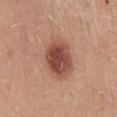Q: Was a biopsy performed?
A: imaged on a skin check; not biopsied
Q: What are the patient's age and sex?
A: male, aged around 25
Q: Lesion size?
A: ≈5 mm
Q: How was the tile lit?
A: white-light illumination
Q: Automated lesion metrics?
A: an average lesion color of about L≈49 a*≈24 b*≈27 (CIELAB), about 14 CIELAB-L* units darker than the surrounding skin, and a normalized lesion–skin contrast near 9.5; a border-irregularity index near 1.5/10, a within-lesion color-variation index near 5.5/10, and a peripheral color-asymmetry measure near 1.5; a nevus-likeness score of about 100/100
Q: Where on the body is the lesion?
A: the mid back
Q: What is the imaging modality?
A: 15 mm crop, total-body photography